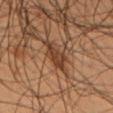This lesion was catalogued during total-body skin photography and was not selected for biopsy.
Located on the left upper arm.
The recorded lesion diameter is about 3.5 mm.
Imaged with cross-polarized lighting.
A male patient aged 53–57.
This image is a 15 mm lesion crop taken from a total-body photograph.